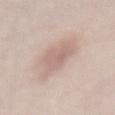Impression:
The lesion was photographed on a routine skin check and not biopsied; there is no pathology result.
Background:
A female subject, aged 48 to 52. The recorded lesion diameter is about 5.5 mm. The lesion-visualizer software estimated an area of roughly 13 mm² and a shape eccentricity near 0.8. The analysis additionally found a mean CIELAB color near L≈65 a*≈16 b*≈24 and a lesion-to-skin contrast of about 5.5 (normalized; higher = more distinct). The software also gave an automated nevus-likeness rating near 30 out of 100 and a lesion-detection confidence of about 95/100. This image is a 15 mm lesion crop taken from a total-body photograph. The tile uses white-light illumination. The lesion is located on the lower back.